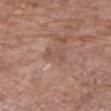notes=total-body-photography surveillance lesion; no biopsy | lesion size=≈3 mm | illumination=white-light illumination | acquisition=~15 mm tile from a whole-body skin photo | body site=the right upper arm | patient=female, aged around 70.